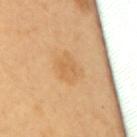| key | value |
|---|---|
| follow-up | total-body-photography surveillance lesion; no biopsy |
| anatomic site | the upper back |
| diameter | about 3.5 mm |
| acquisition | total-body-photography crop, ~15 mm field of view |
| lighting | cross-polarized |
| subject | female, aged 58–62 |
| automated lesion analysis | a border-irregularity index near 2/10, a color-variation rating of about 2/10, and a peripheral color-asymmetry measure near 0.5; a nevus-likeness score of about 10/100 and a lesion-detection confidence of about 100/100 |